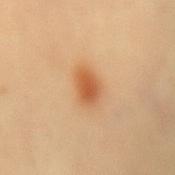The lesion was tiled from a total-body skin photograph and was not biopsied. This is a cross-polarized tile. An algorithmic analysis of the crop reported a mean CIELAB color near L≈57 a*≈25 b*≈41, roughly 11 lightness units darker than nearby skin, and a normalized border contrast of about 8. The lesion is on the lower back. A close-up tile cropped from a whole-body skin photograph, about 15 mm across. The subject is a female in their mid-40s.